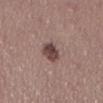The lesion was tiled from a total-body skin photograph and was not biopsied.
Captured under white-light illumination.
A lesion tile, about 15 mm wide, cut from a 3D total-body photograph.
The subject is a male aged 38 to 42.
The lesion is on the abdomen.
Automated image analysis of the tile measured a classifier nevus-likeness of about 65/100.
Longest diameter approximately 3.5 mm.